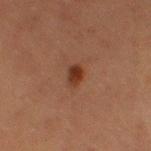No biopsy was performed on this lesion — it was imaged during a full skin examination and was not determined to be concerning. A lesion tile, about 15 mm wide, cut from a 3D total-body photograph. A female subject aged approximately 50. The lesion is on the left thigh. Automated image analysis of the tile measured an automated nevus-likeness rating near 100 out of 100 and a detector confidence of about 100 out of 100 that the crop contains a lesion. Captured under cross-polarized illumination.